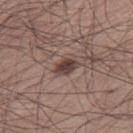The lesion was photographed on a routine skin check and not biopsied; there is no pathology result.
The lesion-visualizer software estimated an outline eccentricity of about 0.8 (0 = round, 1 = elongated) and two-axis asymmetry of about 0.25. And it measured an average lesion color of about L≈40 a*≈18 b*≈20 (CIELAB), roughly 13 lightness units darker than nearby skin, and a normalized border contrast of about 10.5. The software also gave a within-lesion color-variation index near 2.5/10 and peripheral color asymmetry of about 1.
This is a white-light tile.
A male patient, about 30 years old.
The lesion is located on the right thigh.
A 15 mm close-up tile from a total-body photography series done for melanoma screening.